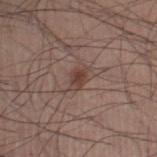biopsy status: total-body-photography surveillance lesion; no biopsy
subject: male, in their mid-30s
acquisition: ~15 mm crop, total-body skin-cancer survey
site: the leg